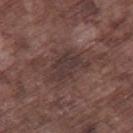Notes:
• notes · imaged on a skin check; not biopsied
• subject · male, roughly 75 years of age
• imaging modality · ~15 mm tile from a whole-body skin photo
• site · the right thigh